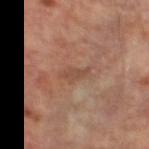| field | value |
|---|---|
| biopsy status | no biopsy performed (imaged during a skin exam) |
| body site | the right lower leg |
| patient | male, aged around 65 |
| image source | total-body-photography crop, ~15 mm field of view |